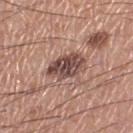| feature | finding |
|---|---|
| workup | imaged on a skin check; not biopsied |
| subject | male, approximately 55 years of age |
| imaging modality | ~15 mm tile from a whole-body skin photo |
| site | the left thigh |
| lighting | white-light |
| size | ~4.5 mm (longest diameter) |